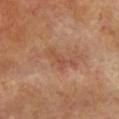Context:
An algorithmic analysis of the crop reported a lesion area of about 2.5 mm², an outline eccentricity of about 0.9 (0 = round, 1 = elongated), and two-axis asymmetry of about 0.4. The software also gave a border-irregularity rating of about 4.5/10, internal color variation of about 0 on a 0–10 scale, and peripheral color asymmetry of about 0. Longest diameter approximately 2.5 mm. A female subject in their mid-70s. A region of skin cropped from a whole-body photographic capture, roughly 15 mm wide. On the upper back. Imaged with cross-polarized lighting.